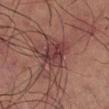Q: Is there a histopathology result?
A: total-body-photography surveillance lesion; no biopsy
Q: What kind of image is this?
A: ~15 mm crop, total-body skin-cancer survey
Q: Who is the patient?
A: male, approximately 60 years of age